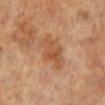  biopsy_status: not biopsied; imaged during a skin examination
  patient:
    sex: female
    age_approx: 65
  lesion_size:
    long_diameter_mm_approx: 5.0
  image:
    source: total-body photography crop
    field_of_view_mm: 15
  site: right leg
  automated_metrics:
    area_mm2_approx: 11.0
    eccentricity: 0.75
    border_irregularity_0_10: 4.0
    peripheral_color_asymmetry: 1.0
    nevus_likeness_0_100: 5